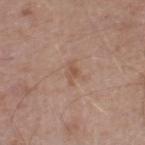Assessment:
Captured during whole-body skin photography for melanoma surveillance; the lesion was not biopsied.
Acquisition and patient details:
Automated tile analysis of the lesion measured a normalized lesion–skin contrast near 5.5. The lesion is on the left thigh. Imaged with white-light lighting. A close-up tile cropped from a whole-body skin photograph, about 15 mm across. A male patient in their mid- to late 60s. Approximately 2.5 mm at its widest.